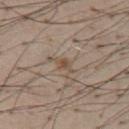notes = total-body-photography surveillance lesion; no biopsy | location = the chest | image = ~15 mm tile from a whole-body skin photo | subject = male, about 55 years old | illumination = white-light | image-analysis metrics = an average lesion color of about L≈50 a*≈14 b*≈27 (CIELAB), about 8 CIELAB-L* units darker than the surrounding skin, and a normalized lesion–skin contrast near 6.5.